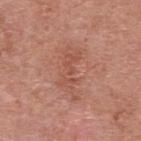  biopsy_status: not biopsied; imaged during a skin examination
  image:
    source: total-body photography crop
    field_of_view_mm: 15
  lesion_size:
    long_diameter_mm_approx: 5.5
  patient:
    sex: female
    age_approx: 70
  site: upper back